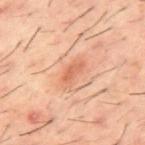Notes:
• biopsy status: catalogued during a skin exam; not biopsied
• location: the mid back
• subject: male, in their 60s
• size: ≈3 mm
• tile lighting: cross-polarized
• acquisition: 15 mm crop, total-body photography